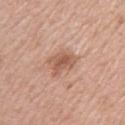{"site": "right upper arm", "patient": {"sex": "male", "age_approx": 55}, "automated_metrics": {"eccentricity": 0.8, "shape_asymmetry": 0.45, "cielab_L": 56, "cielab_a": 22, "cielab_b": 30, "vs_skin_contrast_norm": 7.0}, "lesion_size": {"long_diameter_mm_approx": 3.5}, "lighting": "white-light", "image": {"source": "total-body photography crop", "field_of_view_mm": 15}}